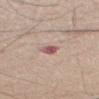biopsy_status: not biopsied; imaged during a skin examination
image:
  source: total-body photography crop
  field_of_view_mm: 15
lesion_size:
  long_diameter_mm_approx: 3.0
patient:
  sex: male
  age_approx: 55
lighting: white-light